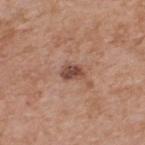follow-up: imaged on a skin check; not biopsied
diameter: about 2.5 mm
subject: male, approximately 60 years of age
location: the upper back
image: ~15 mm crop, total-body skin-cancer survey